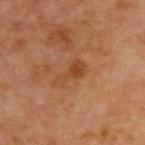follow-up: imaged on a skin check; not biopsied | patient: male, in their mid-60s | body site: the chest | image source: ~15 mm tile from a whole-body skin photo | size: ≈3.5 mm | TBP lesion metrics: a nevus-likeness score of about 0/100 | lighting: cross-polarized illumination.